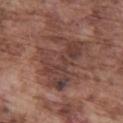Findings:
* workup · imaged on a skin check; not biopsied
* lighting · white-light illumination
* diameter · ≈7 mm
* subject · male, aged around 75
* location · the leg
* imaging modality · ~15 mm crop, total-body skin-cancer survey